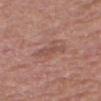Case summary:
– biopsy status · no biopsy performed (imaged during a skin exam)
– image source · ~15 mm tile from a whole-body skin photo
– site · the upper back
– patient · male, in their mid-50s
– lesion diameter · ~3.5 mm (longest diameter)
– automated metrics · an area of roughly 4 mm² and a shape eccentricity near 0.9; a mean CIELAB color near L≈50 a*≈21 b*≈24, roughly 7 lightness units darker than nearby skin, and a normalized border contrast of about 5; an automated nevus-likeness rating near 0 out of 100 and lesion-presence confidence of about 100/100
– lighting · white-light illumination